A 15 mm crop from a total-body photograph taken for skin-cancer surveillance · a female subject, aged 63–67 · the lesion is on the left lower leg:
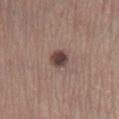Captured under white-light illumination. An algorithmic analysis of the crop reported an automated nevus-likeness rating near 90 out of 100 and a lesion-detection confidence of about 100/100. Measured at roughly 2.5 mm in maximum diameter. Histopathologically confirmed as an atypical intraepithelial melanocytic proliferation — a borderline lesion of uncertain malignant potential.